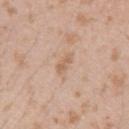This lesion was catalogued during total-body skin photography and was not selected for biopsy.
Located on the left upper arm.
An algorithmic analysis of the crop reported internal color variation of about 0 on a 0–10 scale and radial color variation of about 0.
The tile uses white-light illumination.
A male patient roughly 25 years of age.
A 15 mm crop from a total-body photograph taken for skin-cancer surveillance.
Measured at roughly 2.5 mm in maximum diameter.